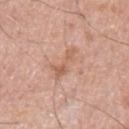The lesion was tiled from a total-body skin photograph and was not biopsied.
Automated image analysis of the tile measured a classifier nevus-likeness of about 0/100 and a detector confidence of about 100 out of 100 that the crop contains a lesion.
The patient is a male about 80 years old.
Cropped from a whole-body photographic skin survey; the tile spans about 15 mm.
From the arm.
About 4 mm across.
The tile uses white-light illumination.